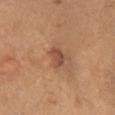{
  "biopsy_status": "not biopsied; imaged during a skin examination",
  "patient": {
    "sex": "female",
    "age_approx": 55
  },
  "site": "left upper arm",
  "automated_metrics": {
    "peripheral_color_asymmetry": 0.5,
    "nevus_likeness_0_100": 40
  },
  "image": {
    "source": "total-body photography crop",
    "field_of_view_mm": 15
  },
  "lesion_size": {
    "long_diameter_mm_approx": 2.5
  },
  "lighting": "white-light"
}